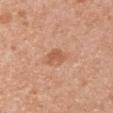This is a white-light tile. A close-up tile cropped from a whole-body skin photograph, about 15 mm across. From the right upper arm. A female patient, about 50 years old.